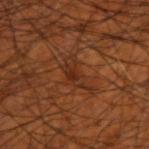{"biopsy_status": "not biopsied; imaged during a skin examination", "site": "left forearm", "patient": {"sex": "male", "age_approx": 70}, "image": {"source": "total-body photography crop", "field_of_view_mm": 15}, "lighting": "cross-polarized"}